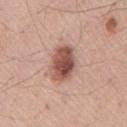biopsy status=total-body-photography surveillance lesion; no biopsy
illumination=white-light
image=total-body-photography crop, ~15 mm field of view
diameter=about 4.5 mm
site=the front of the torso
patient=male, roughly 55 years of age